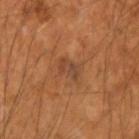workup: total-body-photography surveillance lesion; no biopsy | automated metrics: a footprint of about 4 mm², a shape eccentricity near 0.8, and two-axis asymmetry of about 0.5 | subject: male, aged 48–52 | lighting: cross-polarized illumination | lesion size: about 3 mm | body site: the left forearm | image: ~15 mm tile from a whole-body skin photo.